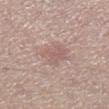A 15 mm close-up extracted from a 3D total-body photography capture.
The lesion is located on the left lower leg.
Automated tile analysis of the lesion measured an area of roughly 8.5 mm², an outline eccentricity of about 0.7 (0 = round, 1 = elongated), and a shape-asymmetry score of about 0.25 (0 = symmetric). And it measured about 7 CIELAB-L* units darker than the surrounding skin and a normalized border contrast of about 4.5. The software also gave an automated nevus-likeness rating near 0 out of 100.
About 4 mm across.
This is a white-light tile.
A male patient, aged around 55.